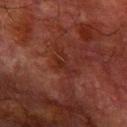Captured during whole-body skin photography for melanoma surveillance; the lesion was not biopsied.
Cropped from a total-body skin-imaging series; the visible field is about 15 mm.
The patient is a male roughly 80 years of age.
The lesion is on the right forearm.